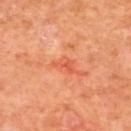site: the upper back
subject: male, in their 70s
tile lighting: cross-polarized illumination
automated metrics: an average lesion color of about L≈58 a*≈36 b*≈40 (CIELAB) and a lesion–skin lightness drop of about 7; a nevus-likeness score of about 0/100 and a detector confidence of about 100 out of 100 that the crop contains a lesion
acquisition: ~15 mm crop, total-body skin-cancer survey